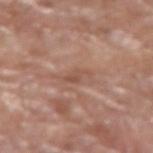| field | value |
|---|---|
| follow-up | imaged on a skin check; not biopsied |
| patient | female, aged 78–82 |
| automated lesion analysis | border irregularity of about 3.5 on a 0–10 scale, internal color variation of about 2 on a 0–10 scale, and a peripheral color-asymmetry measure near 0.5 |
| imaging modality | ~15 mm tile from a whole-body skin photo |
| illumination | white-light |
| site | the right forearm |